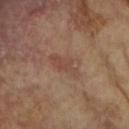Captured during whole-body skin photography for melanoma surveillance; the lesion was not biopsied. About 4 mm across. The tile uses cross-polarized illumination. The lesion is located on the right forearm. Cropped from a whole-body photographic skin survey; the tile spans about 15 mm. The subject is a female in their mid- to late 70s.